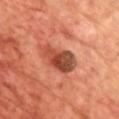<record>
  <site>front of the torso</site>
  <image>
    <source>total-body photography crop</source>
    <field_of_view_mm>15</field_of_view_mm>
  </image>
  <lighting>cross-polarized</lighting>
  <patient>
    <sex>male</sex>
    <age_approx>70</age_approx>
  </patient>
  <automated_metrics>
    <area_mm2_approx>11.0</area_mm2_approx>
    <eccentricity>0.85</eccentricity>
    <shape_asymmetry>0.25</shape_asymmetry>
    <cielab_L>46</cielab_L>
    <cielab_a>29</cielab_a>
    <cielab_b>33</cielab_b>
    <vs_skin_darker_L>13.0</vs_skin_darker_L>
    <vs_skin_contrast_norm>9.5</vs_skin_contrast_norm>
    <nevus_likeness_0_100>30</nevus_likeness_0_100>
    <lesion_detection_confidence_0_100>100</lesion_detection_confidence_0_100>
  </automated_metrics>
  <lesion_size>
    <long_diameter_mm_approx>5.0</long_diameter_mm_approx>
  </lesion_size>
</record>